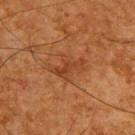notes=catalogued during a skin exam; not biopsied
image source=total-body-photography crop, ~15 mm field of view
patient=male, aged approximately 65
lesion size=about 4 mm
lighting=cross-polarized illumination
body site=the upper back